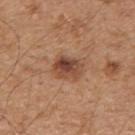patient: male, aged 43–47 | image-analysis metrics: an automated nevus-likeness rating near 85 out of 100 | size: ~3.5 mm (longest diameter) | site: the upper back | tile lighting: white-light | image: total-body-photography crop, ~15 mm field of view.